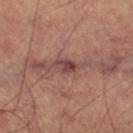Findings:
* biopsy status · no biopsy performed (imaged during a skin exam)
* tile lighting · cross-polarized
* imaging modality · 15 mm crop, total-body photography
* site · the right thigh
* subject · male, aged around 65